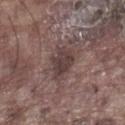biopsy status: no biopsy performed (imaged during a skin exam)
location: the left lower leg
size: ≈4.5 mm
patient: male, in their mid-70s
image-analysis metrics: an average lesion color of about L≈39 a*≈15 b*≈17 (CIELAB) and roughly 10 lightness units darker than nearby skin; a border-irregularity index near 3.5/10, a within-lesion color-variation index near 3/10, and peripheral color asymmetry of about 1; an automated nevus-likeness rating near 5 out of 100 and a lesion-detection confidence of about 95/100
acquisition: ~15 mm crop, total-body skin-cancer survey